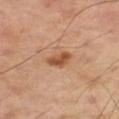Findings:
* notes · catalogued during a skin exam; not biopsied
* acquisition · total-body-photography crop, ~15 mm field of view
* site · the right thigh